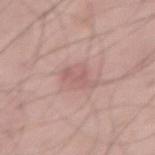Imaged during a routine full-body skin examination; the lesion was not biopsied and no histopathology is available.
A 15 mm crop from a total-body photograph taken for skin-cancer surveillance.
Located on the abdomen.
A male patient, aged 53–57.